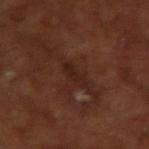biopsy_status: not biopsied; imaged during a skin examination
lesion_size:
  long_diameter_mm_approx: 3.0
image:
  source: total-body photography crop
  field_of_view_mm: 15
site: arm
lighting: cross-polarized
patient:
  sex: male
  age_approx: 65